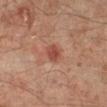Notes:
- workup: catalogued during a skin exam; not biopsied
- acquisition: ~15 mm tile from a whole-body skin photo
- body site: the left leg
- lighting: cross-polarized
- patient: male, roughly 50 years of age
- size: about 2.5 mm